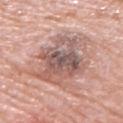Q: Was this lesion biopsied?
A: imaged on a skin check; not biopsied
Q: Where on the body is the lesion?
A: the right upper arm
Q: How was the tile lit?
A: white-light illumination
Q: Who is the patient?
A: male, in their 80s
Q: Lesion size?
A: ≈6.5 mm
Q: What kind of image is this?
A: ~15 mm tile from a whole-body skin photo